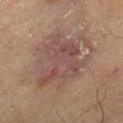{
  "biopsy_status": "not biopsied; imaged during a skin examination",
  "image": {
    "source": "total-body photography crop",
    "field_of_view_mm": 15
  },
  "lesion_size": {
    "long_diameter_mm_approx": 6.5
  },
  "patient": {
    "sex": "male",
    "age_approx": 70
  },
  "site": "left thigh"
}